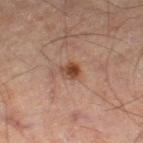Clinical impression: The lesion was tiled from a total-body skin photograph and was not biopsied. Clinical summary: Imaged with cross-polarized lighting. An algorithmic analysis of the crop reported a footprint of about 3.5 mm², an outline eccentricity of about 0.6 (0 = round, 1 = elongated), and a symmetry-axis asymmetry near 0.3. And it measured roughly 11 lightness units darker than nearby skin and a lesion-to-skin contrast of about 9.5 (normalized; higher = more distinct). And it measured a classifier nevus-likeness of about 95/100. A close-up tile cropped from a whole-body skin photograph, about 15 mm across. The lesion is located on the left thigh. The patient is a male aged 63 to 67. Approximately 2.5 mm at its widest.